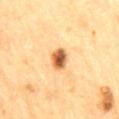Q: Is there a histopathology result?
A: no biopsy performed (imaged during a skin exam)
Q: Where on the body is the lesion?
A: the mid back
Q: Who is the patient?
A: male, roughly 85 years of age or older
Q: How was this image acquired?
A: 15 mm crop, total-body photography